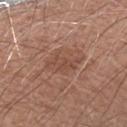No biopsy was performed on this lesion — it was imaged during a full skin examination and was not determined to be concerning.
The lesion is on the arm.
This is a white-light tile.
A male patient aged around 70.
Longest diameter approximately 4 mm.
Cropped from a total-body skin-imaging series; the visible field is about 15 mm.